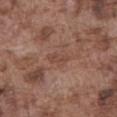Impression: No biopsy was performed on this lesion — it was imaged during a full skin examination and was not determined to be concerning. Context: The lesion-visualizer software estimated an area of roughly 3 mm², a shape eccentricity near 0.9, and two-axis asymmetry of about 0.35. And it measured an average lesion color of about L≈45 a*≈20 b*≈26 (CIELAB) and a lesion–skin lightness drop of about 6. A male patient, aged 73 to 77. On the front of the torso. The recorded lesion diameter is about 3 mm. A 15 mm crop from a total-body photograph taken for skin-cancer surveillance. The tile uses white-light illumination.